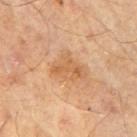Acquisition and patient details:
About 4 mm across. A male subject, aged approximately 65. Cropped from a total-body skin-imaging series; the visible field is about 15 mm. Located on the right upper arm.